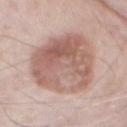Q: What lighting was used for the tile?
A: white-light illumination
Q: What kind of image is this?
A: ~15 mm crop, total-body skin-cancer survey
Q: Who is the patient?
A: male, aged around 85 or older
Q: What is the lesion's diameter?
A: about 9 mm
Q: Automated lesion metrics?
A: a shape eccentricity near 0.7; a border-irregularity index near 2/10, a within-lesion color-variation index near 7/10, and radial color variation of about 2.5
Q: Lesion location?
A: the front of the torso